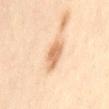location: the lower back | imaging modality: 15 mm crop, total-body photography | TBP lesion metrics: a footprint of about 7.5 mm², a shape eccentricity near 0.8, and two-axis asymmetry of about 0.15 | tile lighting: cross-polarized illumination | subject: female, aged around 40.